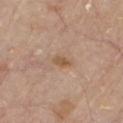{
  "biopsy_status": "not biopsied; imaged during a skin examination",
  "automated_metrics": {
    "vs_skin_darker_L": 8.0,
    "border_irregularity_0_10": 2.5,
    "peripheral_color_asymmetry": 1.0,
    "nevus_likeness_0_100": 5,
    "lesion_detection_confidence_0_100": 100
  },
  "image": {
    "source": "total-body photography crop",
    "field_of_view_mm": 15
  },
  "site": "chest",
  "patient": {
    "sex": "male",
    "age_approx": 80
  },
  "lighting": "white-light"
}